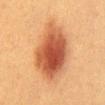<case>
  <biopsy_status>not biopsied; imaged during a skin examination</biopsy_status>
  <image>
    <source>total-body photography crop</source>
    <field_of_view_mm>15</field_of_view_mm>
  </image>
  <site>chest</site>
  <lesion_size>
    <long_diameter_mm_approx>9.5</long_diameter_mm_approx>
  </lesion_size>
  <patient>
    <sex>female</sex>
    <age_approx>40</age_approx>
  </patient>
</case>